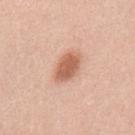Part of a total-body skin-imaging series; this lesion was reviewed on a skin check and was not flagged for biopsy.
Automated image analysis of the tile measured a shape eccentricity near 0.8 and a symmetry-axis asymmetry near 0.2. The software also gave a color-variation rating of about 2.5/10 and peripheral color asymmetry of about 1.
The tile uses white-light illumination.
Located on the back.
The patient is a male aged 43 to 47.
Longest diameter approximately 4 mm.
A region of skin cropped from a whole-body photographic capture, roughly 15 mm wide.